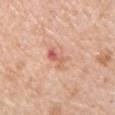Imaged during a routine full-body skin examination; the lesion was not biopsied and no histopathology is available. A 15 mm crop from a total-body photograph taken for skin-cancer surveillance. The subject is a female in their mid-40s. Captured under white-light illumination. The lesion is located on the front of the torso. Measured at roughly 3.5 mm in maximum diameter. An algorithmic analysis of the crop reported a within-lesion color-variation index near 10/10 and peripheral color asymmetry of about 4. The analysis additionally found an automated nevus-likeness rating near 0 out of 100.